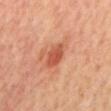biopsy status: imaged on a skin check; not biopsied
patient: male, aged 63 to 67
location: the mid back
imaging modality: 15 mm crop, total-body photography
lesion diameter: ~3.5 mm (longest diameter)
lighting: cross-polarized